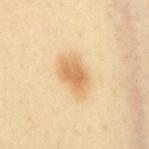biopsy status: catalogued during a skin exam; not biopsied
image: 15 mm crop, total-body photography
location: the mid back
TBP lesion metrics: a lesion area of about 9.5 mm², an outline eccentricity of about 0.8 (0 = round, 1 = elongated), and a shape-asymmetry score of about 0.2 (0 = symmetric); a border-irregularity rating of about 2/10, a color-variation rating of about 4/10, and radial color variation of about 1
subject: female, aged 38 to 42